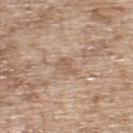Part of a total-body skin-imaging series; this lesion was reviewed on a skin check and was not flagged for biopsy. Captured under white-light illumination. Located on the upper back. A male subject roughly 65 years of age. About 3 mm across. A 15 mm close-up extracted from a 3D total-body photography capture.